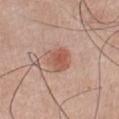The lesion was photographed on a routine skin check and not biopsied; there is no pathology result.
Cropped from a whole-body photographic skin survey; the tile spans about 15 mm.
Measured at roughly 3.5 mm in maximum diameter.
Located on the chest.
This is a white-light tile.
The total-body-photography lesion software estimated an area of roughly 7.5 mm², an outline eccentricity of about 0.5 (0 = round, 1 = elongated), and a shape-asymmetry score of about 0.2 (0 = symmetric). The analysis additionally found a mean CIELAB color near L≈56 a*≈24 b*≈29, about 10 CIELAB-L* units darker than the surrounding skin, and a normalized border contrast of about 7. The analysis additionally found an automated nevus-likeness rating near 100 out of 100 and lesion-presence confidence of about 100/100.
A male patient in their mid- to late 70s.